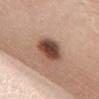Impression: Part of a total-body skin-imaging series; this lesion was reviewed on a skin check and was not flagged for biopsy. Context: The lesion is on the chest. A 15 mm close-up extracted from a 3D total-body photography capture. The lesion's longest dimension is about 4.5 mm. The total-body-photography lesion software estimated a footprint of about 10 mm², an outline eccentricity of about 0.75 (0 = round, 1 = elongated), and a shape-asymmetry score of about 0.2 (0 = symmetric). It also reported internal color variation of about 6 on a 0–10 scale. The tile uses white-light illumination. A female subject, approximately 50 years of age.